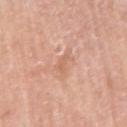| key | value |
|---|---|
| acquisition | 15 mm crop, total-body photography |
| illumination | white-light |
| anatomic site | the right upper arm |
| patient | male, about 60 years old |